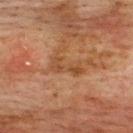Part of a total-body skin-imaging series; this lesion was reviewed on a skin check and was not flagged for biopsy. Captured under cross-polarized illumination. The lesion is located on the upper back. Cropped from a whole-body photographic skin survey; the tile spans about 15 mm. Measured at roughly 4 mm in maximum diameter. The subject is a male aged around 75.